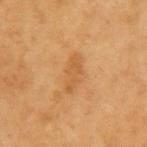No biopsy was performed on this lesion — it was imaged during a full skin examination and was not determined to be concerning. Longest diameter approximately 4 mm. Imaged with cross-polarized lighting. A male subject roughly 60 years of age. A roughly 15 mm field-of-view crop from a total-body skin photograph. The lesion is on the left upper arm. An algorithmic analysis of the crop reported a lesion area of about 6 mm², an outline eccentricity of about 0.9 (0 = round, 1 = elongated), and a symmetry-axis asymmetry near 0.25. It also reported about 7 CIELAB-L* units darker than the surrounding skin and a normalized border contrast of about 5.5. It also reported internal color variation of about 1.5 on a 0–10 scale.